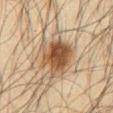| field | value |
|---|---|
| notes | total-body-photography surveillance lesion; no biopsy |
| image | ~15 mm crop, total-body skin-cancer survey |
| patient | male, aged around 65 |
| lesion size | ≈5 mm |
| illumination | cross-polarized |
| anatomic site | the abdomen |